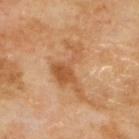The lesion was tiled from a total-body skin photograph and was not biopsied.
A lesion tile, about 15 mm wide, cut from a 3D total-body photograph.
A male patient, aged 68–72.
The lesion is located on the back.
Captured under cross-polarized illumination.
Approximately 7 mm at its widest.